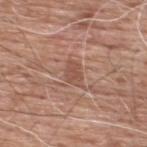Clinical impression: The lesion was photographed on a routine skin check and not biopsied; there is no pathology result. Clinical summary: Automated image analysis of the tile measured an average lesion color of about L≈51 a*≈21 b*≈27 (CIELAB) and a normalized border contrast of about 6. A male patient in their 60s. The lesion is located on the upper back. A lesion tile, about 15 mm wide, cut from a 3D total-body photograph.